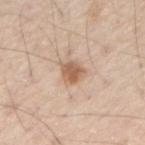{
  "biopsy_status": "not biopsied; imaged during a skin examination",
  "patient": {
    "sex": "male",
    "age_approx": 60
  },
  "site": "right forearm",
  "lesion_size": {
    "long_diameter_mm_approx": 2.5
  },
  "image": {
    "source": "total-body photography crop",
    "field_of_view_mm": 15
  },
  "automated_metrics": {
    "area_mm2_approx": 5.0,
    "shape_asymmetry": 0.2,
    "border_irregularity_0_10": 2.0,
    "color_variation_0_10": 2.5,
    "peripheral_color_asymmetry": 1.0
  },
  "lighting": "white-light"
}